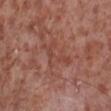workup: catalogued during a skin exam; not biopsied | subject: male, in their mid- to late 50s | imaging modality: ~15 mm crop, total-body skin-cancer survey | lesion size: about 5 mm | illumination: white-light illumination | location: the left lower leg.